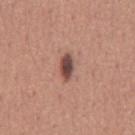Part of a total-body skin-imaging series; this lesion was reviewed on a skin check and was not flagged for biopsy.